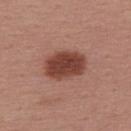  biopsy_status: not biopsied; imaged during a skin examination
  patient:
    sex: female
    age_approx: 55
  automated_metrics:
    area_mm2_approx: 14.0
    eccentricity: 0.75
    vs_skin_darker_L: 14.0
    vs_skin_contrast_norm: 10.5
    color_variation_0_10: 3.5
    peripheral_color_asymmetry: 1.0
  lighting: white-light
  lesion_size:
    long_diameter_mm_approx: 5.0
  image:
    source: total-body photography crop
    field_of_view_mm: 15
  site: upper back